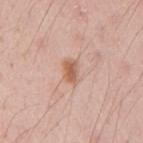The lesion is located on the right upper arm.
Captured under white-light illumination.
A roughly 15 mm field-of-view crop from a total-body skin photograph.
The subject is a male in their 30s.